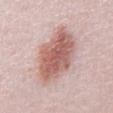No biopsy was performed on this lesion — it was imaged during a full skin examination and was not determined to be concerning. On the chest. The tile uses white-light illumination. A male patient approximately 25 years of age. A 15 mm close-up tile from a total-body photography series done for melanoma screening. The recorded lesion diameter is about 8.5 mm.